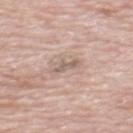Impression:
This lesion was catalogued during total-body skin photography and was not selected for biopsy.
Acquisition and patient details:
An algorithmic analysis of the crop reported a border-irregularity index near 5.5/10 and peripheral color asymmetry of about 0. The analysis additionally found a lesion-detection confidence of about 95/100. The lesion is located on the mid back. Imaged with white-light lighting. Measured at roughly 2.5 mm in maximum diameter. A male subject aged 68 to 72. Cropped from a total-body skin-imaging series; the visible field is about 15 mm.